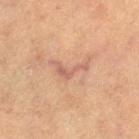notes: no biopsy performed (imaged during a skin exam)
site: the left thigh
lesion size: about 4 mm
lighting: cross-polarized illumination
patient: female, aged 58–62
image: ~15 mm crop, total-body skin-cancer survey